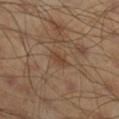Part of a total-body skin-imaging series; this lesion was reviewed on a skin check and was not flagged for biopsy. The patient is a male in their mid-40s. This image is a 15 mm lesion crop taken from a total-body photograph. Automated tile analysis of the lesion measured an automated nevus-likeness rating near 5 out of 100 and lesion-presence confidence of about 100/100. The lesion is on the right lower leg. About 2.5 mm across. Imaged with cross-polarized lighting.